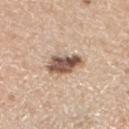  biopsy_status: not biopsied; imaged during a skin examination
  image:
    source: total-body photography crop
    field_of_view_mm: 15
  patient:
    sex: female
    age_approx: 70
  site: left lower leg